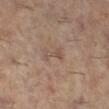Q: Was this lesion biopsied?
A: no biopsy performed (imaged during a skin exam)
Q: What did automated image analysis measure?
A: an automated nevus-likeness rating near 0 out of 100 and a detector confidence of about 100 out of 100 that the crop contains a lesion
Q: What is the imaging modality?
A: total-body-photography crop, ~15 mm field of view
Q: Illumination type?
A: cross-polarized illumination
Q: Who is the patient?
A: female, aged 58–62
Q: What is the lesion's diameter?
A: ~2.5 mm (longest diameter)
Q: What is the anatomic site?
A: the right lower leg